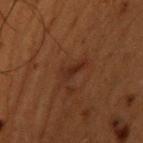The lesion was tiled from a total-body skin photograph and was not biopsied. From the upper back. Imaged with cross-polarized lighting. The subject is a male in their 50s. A close-up tile cropped from a whole-body skin photograph, about 15 mm across. Longest diameter approximately 3 mm.